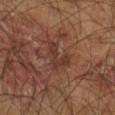Background:
Longest diameter approximately 4 mm. A male subject, aged 58 to 62. From the right leg. The lesion-visualizer software estimated a lesion area of about 5.5 mm², a shape eccentricity near 0.85, and a symmetry-axis asymmetry near 0.65. The software also gave a mean CIELAB color near L≈33 a*≈20 b*≈24, about 7 CIELAB-L* units darker than the surrounding skin, and a lesion-to-skin contrast of about 6.5 (normalized; higher = more distinct). This is a cross-polarized tile. A 15 mm crop from a total-body photograph taken for skin-cancer surveillance.